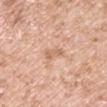| field | value |
|---|---|
| follow-up | imaged on a skin check; not biopsied |
| imaging modality | ~15 mm tile from a whole-body skin photo |
| illumination | white-light |
| body site | the right upper arm |
| automated metrics | an area of roughly 2.5 mm², an outline eccentricity of about 0.9 (0 = round, 1 = elongated), and two-axis asymmetry of about 0.4; a border-irregularity rating of about 4.5/10, internal color variation of about 0 on a 0–10 scale, and peripheral color asymmetry of about 0; an automated nevus-likeness rating near 0 out of 100 and lesion-presence confidence of about 100/100 |
| subject | male, in their mid- to late 50s |
| lesion size | ~2.5 mm (longest diameter) |